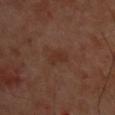size: ≈3 mm | subject: male, aged approximately 50 | body site: the back | image source: 15 mm crop, total-body photography | lighting: cross-polarized | image-analysis metrics: a border-irregularity rating of about 3.5/10, a within-lesion color-variation index near 1/10, and a peripheral color-asymmetry measure near 0.5; a lesion-detection confidence of about 100/100.